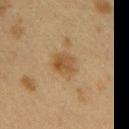A female subject about 40 years old. The tile uses cross-polarized illumination. Measured at roughly 3 mm in maximum diameter. From the right upper arm. A close-up tile cropped from a whole-body skin photograph, about 15 mm across.